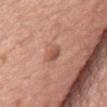follow-up = no biopsy performed (imaged during a skin exam); lighting = white-light illumination; diameter = ~3 mm (longest diameter); image = ~15 mm tile from a whole-body skin photo; TBP lesion metrics = a border-irregularity rating of about 2.5/10, a color-variation rating of about 2/10, and peripheral color asymmetry of about 0.5; site = the mid back; patient = male, aged approximately 75.